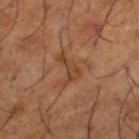No biopsy was performed on this lesion — it was imaged during a full skin examination and was not determined to be concerning. A male subject, aged approximately 65. This is a cross-polarized tile. The lesion's longest dimension is about 4 mm. A 15 mm crop from a total-body photograph taken for skin-cancer surveillance. The total-body-photography lesion software estimated an area of roughly 5 mm², an outline eccentricity of about 0.85 (0 = round, 1 = elongated), and a shape-asymmetry score of about 0.6 (0 = symmetric). And it measured an average lesion color of about L≈43 a*≈22 b*≈34 (CIELAB), roughly 7 lightness units darker than nearby skin, and a normalized border contrast of about 6. The software also gave a classifier nevus-likeness of about 0/100 and lesion-presence confidence of about 100/100. On the leg.